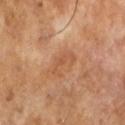A male patient about 65 years old.
A close-up tile cropped from a whole-body skin photograph, about 15 mm across.